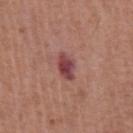Case summary:
– tile lighting — white-light
– anatomic site — the chest
– patient — male, aged around 65
– acquisition — total-body-photography crop, ~15 mm field of view
– lesion diameter — ~3.5 mm (longest diameter)